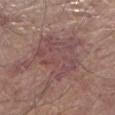<lesion>
<biopsy_status>not biopsied; imaged during a skin examination</biopsy_status>
<image>
  <source>total-body photography crop</source>
  <field_of_view_mm>15</field_of_view_mm>
</image>
<site>left lower leg</site>
<patient>
  <sex>male</sex>
  <age_approx>80</age_approx>
</patient>
</lesion>